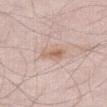Background:
The lesion is located on the leg. A male patient roughly 45 years of age. Approximately 3 mm at its widest. A lesion tile, about 15 mm wide, cut from a 3D total-body photograph. Captured under white-light illumination.